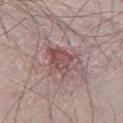Q: What is the imaging modality?
A: 15 mm crop, total-body photography
Q: Where on the body is the lesion?
A: the leg
Q: What lighting was used for the tile?
A: white-light
Q: What is the lesion's diameter?
A: ≈4.5 mm
Q: Who is the patient?
A: male, in their 70s
Q: What did automated image analysis measure?
A: an average lesion color of about L≈51 a*≈21 b*≈19 (CIELAB), roughly 9 lightness units darker than nearby skin, and a normalized lesion–skin contrast near 7